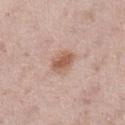Cropped from a total-body skin-imaging series; the visible field is about 15 mm.
A female patient roughly 40 years of age.
Approximately 3 mm at its widest.
From the leg.
The total-body-photography lesion software estimated a footprint of about 6.5 mm², an eccentricity of roughly 0.6, and a shape-asymmetry score of about 0.2 (0 = symmetric). And it measured border irregularity of about 2 on a 0–10 scale, internal color variation of about 3.5 on a 0–10 scale, and radial color variation of about 1. It also reported an automated nevus-likeness rating near 85 out of 100 and lesion-presence confidence of about 100/100.
This is a white-light tile.